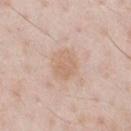Notes:
* illumination — white-light illumination
* subject — male, in their 60s
* acquisition — 15 mm crop, total-body photography
* location — the chest
* image-analysis metrics — an outline eccentricity of about 0.65 (0 = round, 1 = elongated) and a symmetry-axis asymmetry near 0.25; a lesion-detection confidence of about 100/100
* lesion diameter — ≈3.5 mm